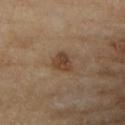This lesion was catalogued during total-body skin photography and was not selected for biopsy.